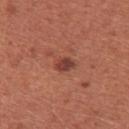Automated image analysis of the tile measured a footprint of about 3.5 mm², a shape eccentricity near 0.8, and a shape-asymmetry score of about 0.25 (0 = symmetric). The software also gave a mean CIELAB color near L≈42 a*≈27 b*≈27 and a normalized lesion–skin contrast near 9. And it measured a border-irregularity index near 2/10, a within-lesion color-variation index near 3.5/10, and peripheral color asymmetry of about 1.
A female patient about 35 years old.
This is a white-light tile.
A 15 mm close-up tile from a total-body photography series done for melanoma screening.
Approximately 2.5 mm at its widest.
The lesion is located on the left upper arm.